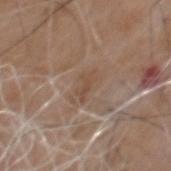Findings:
– follow-up — no biopsy performed (imaged during a skin exam)
– anatomic site — the chest
– lesion size — about 3.5 mm
– subject — male, aged 68 to 72
– image source — ~15 mm tile from a whole-body skin photo